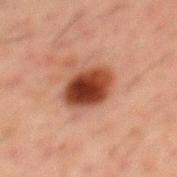No biopsy was performed on this lesion — it was imaged during a full skin examination and was not determined to be concerning. The subject is a male aged around 50. A 15 mm close-up extracted from a 3D total-body photography capture. Approximately 4.5 mm at its widest. This is a cross-polarized tile. From the mid back. The lesion-visualizer software estimated a lesion area of about 12 mm², an outline eccentricity of about 0.7 (0 = round, 1 = elongated), and a symmetry-axis asymmetry near 0.1. The software also gave a mean CIELAB color near L≈33 a*≈23 b*≈27.